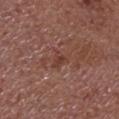Findings:
• biopsy status · catalogued during a skin exam; not biopsied
• body site · the head or neck
• patient · male, aged 68 to 72
• acquisition · total-body-photography crop, ~15 mm field of view